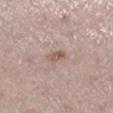Notes:
• follow-up · imaged on a skin check; not biopsied
• lesion size · ≈2.5 mm
• illumination · white-light
• subject · male, aged 58 to 62
• automated metrics · an eccentricity of roughly 0.9 and a shape-asymmetry score of about 0.3 (0 = symmetric); a lesion color around L≈56 a*≈17 b*≈26 in CIELAB and a lesion-to-skin contrast of about 7.5 (normalized; higher = more distinct); a border-irregularity index near 2.5/10 and a peripheral color-asymmetry measure near 0.5
• anatomic site · the left lower leg
• image source · ~15 mm tile from a whole-body skin photo